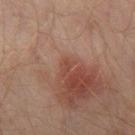Assessment:
Imaged during a routine full-body skin examination; the lesion was not biopsied and no histopathology is available.
Image and clinical context:
Captured under cross-polarized illumination. Approximately 1 mm at its widest. Located on the left leg. A male subject in their 30s. Cropped from a whole-body photographic skin survey; the tile spans about 15 mm.